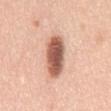This lesion was catalogued during total-body skin photography and was not selected for biopsy.
Approximately 6 mm at its widest.
A male subject aged approximately 50.
Imaged with white-light lighting.
This image is a 15 mm lesion crop taken from a total-body photograph.
The lesion is on the front of the torso.